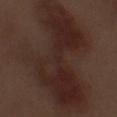subject = male, aged approximately 70; location = the left forearm; image source = ~15 mm crop, total-body skin-cancer survey.